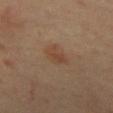The lesion was photographed on a routine skin check and not biopsied; there is no pathology result.
The total-body-photography lesion software estimated a shape eccentricity near 0.8 and a symmetry-axis asymmetry near 0.3. The software also gave a lesion color around L≈41 a*≈17 b*≈28 in CIELAB, about 6 CIELAB-L* units darker than the surrounding skin, and a normalized border contrast of about 6. The software also gave a border-irregularity rating of about 3/10, internal color variation of about 2 on a 0–10 scale, and a peripheral color-asymmetry measure near 0.5.
From the abdomen.
The patient is a male aged 68 to 72.
Measured at roughly 3.5 mm in maximum diameter.
The tile uses cross-polarized illumination.
A 15 mm close-up extracted from a 3D total-body photography capture.